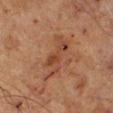site: the right lower leg; imaging modality: ~15 mm tile from a whole-body skin photo; patient: male, aged 68 to 72.